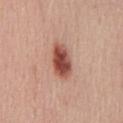Part of a total-body skin-imaging series; this lesion was reviewed on a skin check and was not flagged for biopsy.
A roughly 15 mm field-of-view crop from a total-body skin photograph.
Automated image analysis of the tile measured a nevus-likeness score of about 100/100 and a lesion-detection confidence of about 100/100.
The subject is a male aged 48 to 52.
The tile uses white-light illumination.
Longest diameter approximately 4.5 mm.
On the front of the torso.